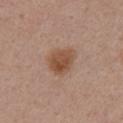{"biopsy_status": "not biopsied; imaged during a skin examination", "site": "right upper arm", "image": {"source": "total-body photography crop", "field_of_view_mm": 15}, "patient": {"sex": "female", "age_approx": 55}}